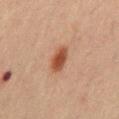<case>
  <biopsy_status>not biopsied; imaged during a skin examination</biopsy_status>
  <lighting>cross-polarized</lighting>
  <image>
    <source>total-body photography crop</source>
    <field_of_view_mm>15</field_of_view_mm>
  </image>
  <automated_metrics>
    <eccentricity>0.8</eccentricity>
    <cielab_L>41</cielab_L>
    <cielab_a>22</cielab_a>
    <cielab_b>28</cielab_b>
    <vs_skin_darker_L>11.0</vs_skin_darker_L>
    <border_irregularity_0_10>1.5</border_irregularity_0_10>
    <color_variation_0_10>3.0</color_variation_0_10>
    <peripheral_color_asymmetry>1.0</peripheral_color_asymmetry>
  </automated_metrics>
  <patient>
    <sex>male</sex>
    <age_approx>70</age_approx>
  </patient>
  <site>abdomen</site>
  <lesion_size>
    <long_diameter_mm_approx>3.0</long_diameter_mm_approx>
  </lesion_size>
</case>